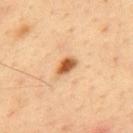Imaged during a routine full-body skin examination; the lesion was not biopsied and no histopathology is available.
From the mid back.
The lesion-visualizer software estimated internal color variation of about 4.5 on a 0–10 scale. The software also gave an automated nevus-likeness rating near 100 out of 100 and a lesion-detection confidence of about 100/100.
Approximately 2.5 mm at its widest.
Cropped from a whole-body photographic skin survey; the tile spans about 15 mm.
A male subject, in their mid- to late 30s.